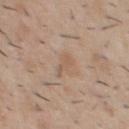{"biopsy_status": "not biopsied; imaged during a skin examination", "lesion_size": {"long_diameter_mm_approx": 3.5}, "site": "chest", "automated_metrics": {"area_mm2_approx": 3.5, "eccentricity": 0.95, "shape_asymmetry": 0.45, "vs_skin_darker_L": 6.0, "vs_skin_contrast_norm": 4.5, "lesion_detection_confidence_0_100": 100}, "image": {"source": "total-body photography crop", "field_of_view_mm": 15}, "lighting": "white-light", "patient": {"sex": "male", "age_approx": 30}}